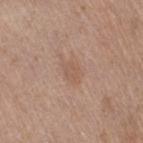Acquisition and patient details:
On the right thigh. Automated image analysis of the tile measured a lesion area of about 4 mm², a shape eccentricity near 0.7, and two-axis asymmetry of about 0.3. The software also gave an average lesion color of about L≈56 a*≈18 b*≈28 (CIELAB) and about 6 CIELAB-L* units darker than the surrounding skin. It also reported a nevus-likeness score of about 0/100 and lesion-presence confidence of about 100/100. Captured under white-light illumination. A female patient, in their 40s. Cropped from a whole-body photographic skin survey; the tile spans about 15 mm. The recorded lesion diameter is about 3 mm.